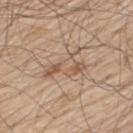The tile uses white-light illumination. About 5 mm across. A 15 mm close-up tile from a total-body photography series done for melanoma screening. A male patient, aged approximately 70. The lesion is on the upper back.